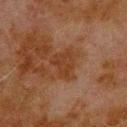{
  "biopsy_status": "not biopsied; imaged during a skin examination",
  "site": "back",
  "lighting": "cross-polarized",
  "patient": {
    "sex": "male",
    "age_approx": 80
  },
  "image": {
    "source": "total-body photography crop",
    "field_of_view_mm": 15
  },
  "lesion_size": {
    "long_diameter_mm_approx": 3.5
  }
}